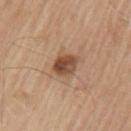Q: Was this lesion biopsied?
A: catalogued during a skin exam; not biopsied
Q: Lesion size?
A: ≈3.5 mm
Q: What lighting was used for the tile?
A: white-light
Q: How was this image acquired?
A: 15 mm crop, total-body photography
Q: Patient demographics?
A: male, in their 80s
Q: What is the anatomic site?
A: the left upper arm
Q: Automated lesion metrics?
A: a lesion area of about 6.5 mm² and an eccentricity of roughly 0.75; a lesion-to-skin contrast of about 10 (normalized; higher = more distinct)